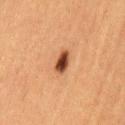Part of a total-body skin-imaging series; this lesion was reviewed on a skin check and was not flagged for biopsy.
Cropped from a whole-body photographic skin survey; the tile spans about 15 mm.
A female subject roughly 40 years of age.
On the left thigh.